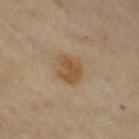image — 15 mm crop, total-body photography
anatomic site — the right upper arm
size — about 4 mm
subject — female, roughly 60 years of age
automated lesion analysis — a lesion area of about 8.5 mm², an outline eccentricity of about 0.65 (0 = round, 1 = elongated), and a symmetry-axis asymmetry near 0.15
tile lighting — cross-polarized